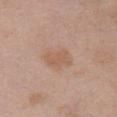The lesion is on the chest. The total-body-photography lesion software estimated a footprint of about 6.5 mm², an eccentricity of roughly 0.75, and a shape-asymmetry score of about 0.35 (0 = symmetric). The software also gave a lesion color around L≈58 a*≈19 b*≈30 in CIELAB and roughly 7 lightness units darker than nearby skin. And it measured internal color variation of about 1.5 on a 0–10 scale and radial color variation of about 0.5. And it measured a classifier nevus-likeness of about 5/100 and a lesion-detection confidence of about 100/100. A 15 mm crop from a total-body photograph taken for skin-cancer surveillance. This is a white-light tile. About 3.5 mm across. A female subject, aged around 60.